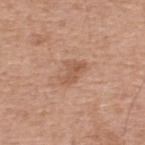Q: Is there a histopathology result?
A: no biopsy performed (imaged during a skin exam)
Q: Who is the patient?
A: male, aged 53–57
Q: What is the anatomic site?
A: the upper back
Q: What did automated image analysis measure?
A: a mean CIELAB color near L≈55 a*≈21 b*≈32 and a normalized border contrast of about 6; a border-irregularity index near 5/10, a within-lesion color-variation index near 2.5/10, and a peripheral color-asymmetry measure near 1; a classifier nevus-likeness of about 20/100 and lesion-presence confidence of about 100/100
Q: Illumination type?
A: white-light illumination
Q: Lesion size?
A: ≈3.5 mm
Q: How was this image acquired?
A: total-body-photography crop, ~15 mm field of view